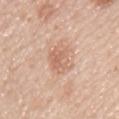No biopsy was performed on this lesion — it was imaged during a full skin examination and was not determined to be concerning.
A 15 mm close-up tile from a total-body photography series done for melanoma screening.
Measured at roughly 3.5 mm in maximum diameter.
From the upper back.
This is a white-light tile.
The total-body-photography lesion software estimated peripheral color asymmetry of about 1. The analysis additionally found a nevus-likeness score of about 0/100 and a lesion-detection confidence of about 100/100.
A male subject about 45 years old.